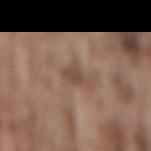{
  "biopsy_status": "not biopsied; imaged during a skin examination",
  "patient": {
    "sex": "male",
    "age_approx": 75
  },
  "lesion_size": {
    "long_diameter_mm_approx": 3.0
  },
  "lighting": "white-light",
  "image": {
    "source": "total-body photography crop",
    "field_of_view_mm": 15
  },
  "automated_metrics": {
    "area_mm2_approx": 4.5,
    "eccentricity": 0.8,
    "shape_asymmetry": 0.4,
    "nevus_likeness_0_100": 0
  },
  "site": "lower back"
}